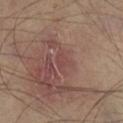No biopsy was performed on this lesion — it was imaged during a full skin examination and was not determined to be concerning.
The lesion-visualizer software estimated an outline eccentricity of about 0.8 (0 = round, 1 = elongated) and two-axis asymmetry of about 0.45.
A male subject aged approximately 65.
The lesion's longest dimension is about 3 mm.
From the right lower leg.
A lesion tile, about 15 mm wide, cut from a 3D total-body photograph.